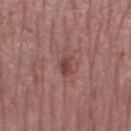biopsy status: total-body-photography surveillance lesion; no biopsy
tile lighting: white-light illumination
patient: female, aged around 65
image source: 15 mm crop, total-body photography
body site: the right thigh
automated metrics: a color-variation rating of about 1.5/10; a classifier nevus-likeness of about 5/100 and a lesion-detection confidence of about 100/100